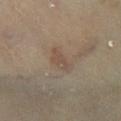Recorded during total-body skin imaging; not selected for excision or biopsy.
This is a cross-polarized tile.
The patient is a female aged 58–62.
The total-body-photography lesion software estimated a lesion color around L≈43 a*≈12 b*≈23 in CIELAB, roughly 5 lightness units darker than nearby skin, and a lesion-to-skin contrast of about 4.5 (normalized; higher = more distinct). And it measured an automated nevus-likeness rating near 5 out of 100.
On the right lower leg.
A lesion tile, about 15 mm wide, cut from a 3D total-body photograph.
Longest diameter approximately 3.5 mm.